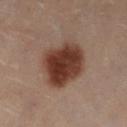This lesion was catalogued during total-body skin photography and was not selected for biopsy. This is a cross-polarized tile. From the leg. A lesion tile, about 15 mm wide, cut from a 3D total-body photograph. A female patient aged 48–52.